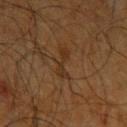The patient is a male about 65 years old. From the left upper arm. Imaged with cross-polarized lighting. A 15 mm close-up tile from a total-body photography series done for melanoma screening. Automated image analysis of the tile measured a mean CIELAB color near L≈25 a*≈15 b*≈26 and a normalized border contrast of about 6. And it measured a border-irregularity index near 6/10, a within-lesion color-variation index near 1.5/10, and a peripheral color-asymmetry measure near 0.5. It also reported lesion-presence confidence of about 90/100. The recorded lesion diameter is about 3.5 mm.